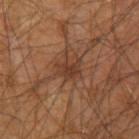Recorded during total-body skin imaging; not selected for excision or biopsy. The lesion-visualizer software estimated a mean CIELAB color near L≈35 a*≈20 b*≈28, a lesion–skin lightness drop of about 7, and a lesion-to-skin contrast of about 7 (normalized; higher = more distinct). The analysis additionally found a classifier nevus-likeness of about 20/100. A male subject about 65 years old. A 15 mm close-up extracted from a 3D total-body photography capture. The lesion is located on the left upper arm. Approximately 3 mm at its widest. The tile uses cross-polarized illumination.